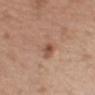notes: imaged on a skin check; not biopsied
patient: male, in their mid-50s
automated metrics: a footprint of about 6.5 mm², an outline eccentricity of about 0.6 (0 = round, 1 = elongated), and a shape-asymmetry score of about 0.25 (0 = symmetric); a classifier nevus-likeness of about 35/100 and a detector confidence of about 100 out of 100 that the crop contains a lesion
image: total-body-photography crop, ~15 mm field of view
lesion diameter: ~3.5 mm (longest diameter)
body site: the left upper arm